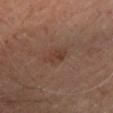The lesion was photographed on a routine skin check and not biopsied; there is no pathology result. A 15 mm close-up tile from a total-body photography series done for melanoma screening. The lesion is located on the right leg. A male subject, aged 58 to 62. Automated tile analysis of the lesion measured a footprint of about 4.5 mm² and a symmetry-axis asymmetry near 0.25. The software also gave an average lesion color of about L≈36 a*≈18 b*≈24 (CIELAB), a lesion–skin lightness drop of about 6, and a lesion-to-skin contrast of about 5.5 (normalized; higher = more distinct). It also reported a classifier nevus-likeness of about 45/100. The recorded lesion diameter is about 3 mm.